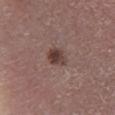follow-up: total-body-photography surveillance lesion; no biopsy | tile lighting: white-light illumination | body site: the leg | acquisition: ~15 mm crop, total-body skin-cancer survey | automated metrics: an area of roughly 5 mm²; a normalized lesion–skin contrast near 9; a border-irregularity index near 2.5/10, a color-variation rating of about 5.5/10, and radial color variation of about 2 | patient: male, approximately 65 years of age.